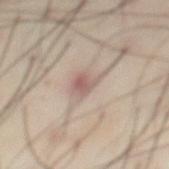Clinical impression: Part of a total-body skin-imaging series; this lesion was reviewed on a skin check and was not flagged for biopsy. Image and clinical context: Cropped from a whole-body photographic skin survey; the tile spans about 15 mm. The lesion is located on the front of the torso. The subject is a male aged approximately 55.